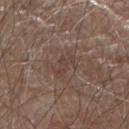The lesion was tiled from a total-body skin photograph and was not biopsied.